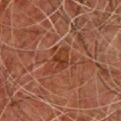Notes:
* follow-up · total-body-photography surveillance lesion; no biopsy